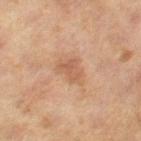This lesion was catalogued during total-body skin photography and was not selected for biopsy. About 3.5 mm across. The lesion is on the right thigh. A female patient about 60 years old. The tile uses cross-polarized illumination. A lesion tile, about 15 mm wide, cut from a 3D total-body photograph.